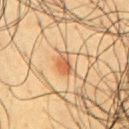Q: Is there a histopathology result?
A: catalogued during a skin exam; not biopsied
Q: How large is the lesion?
A: ≈2.5 mm
Q: Lesion location?
A: the chest
Q: What lighting was used for the tile?
A: cross-polarized
Q: How was this image acquired?
A: ~15 mm crop, total-body skin-cancer survey
Q: Patient demographics?
A: male, roughly 60 years of age